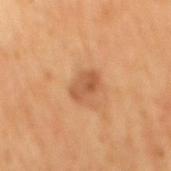biopsy status: catalogued during a skin exam; not biopsied | patient: male, aged 63 to 67 | acquisition: total-body-photography crop, ~15 mm field of view | TBP lesion metrics: an area of roughly 5 mm², an outline eccentricity of about 0.8 (0 = round, 1 = elongated), and a shape-asymmetry score of about 0.25 (0 = symmetric) | body site: the mid back | lighting: cross-polarized | size: about 3 mm.